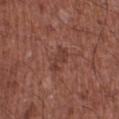Recorded during total-body skin imaging; not selected for excision or biopsy. A 15 mm close-up extracted from a 3D total-body photography capture. On the right lower leg. The recorded lesion diameter is about 3 mm. Captured under white-light illumination. Automated image analysis of the tile measured a mean CIELAB color near L≈39 a*≈23 b*≈25, roughly 7 lightness units darker than nearby skin, and a lesion-to-skin contrast of about 6 (normalized; higher = more distinct). It also reported a within-lesion color-variation index near 0.5/10 and peripheral color asymmetry of about 0. The subject is a male aged 63 to 67.